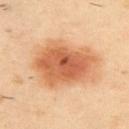Image and clinical context: A lesion tile, about 15 mm wide, cut from a 3D total-body photograph. A male patient, about 40 years old. Longest diameter approximately 8 mm. The total-body-photography lesion software estimated a lesion color around L≈61 a*≈25 b*≈39 in CIELAB, about 14 CIELAB-L* units darker than the surrounding skin, and a lesion-to-skin contrast of about 9 (normalized; higher = more distinct). And it measured a border-irregularity index near 3/10 and a peripheral color-asymmetry measure near 2. The analysis additionally found an automated nevus-likeness rating near 100 out of 100 and a detector confidence of about 100 out of 100 that the crop contains a lesion. On the upper back. Imaged with cross-polarized lighting.